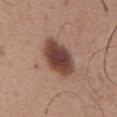Impression:
The lesion was photographed on a routine skin check and not biopsied; there is no pathology result.
Acquisition and patient details:
Approximately 5 mm at its widest. A male subject, aged 63 to 67. This is a white-light tile. The total-body-photography lesion software estimated an area of roughly 14 mm², an eccentricity of roughly 0.75, and a shape-asymmetry score of about 0.1 (0 = symmetric). And it measured a border-irregularity index near 1.5/10, a within-lesion color-variation index near 5/10, and a peripheral color-asymmetry measure near 1.5. A roughly 15 mm field-of-view crop from a total-body skin photograph. From the abdomen.